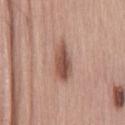The lesion was photographed on a routine skin check and not biopsied; there is no pathology result. From the lower back. The tile uses white-light illumination. The patient is a male about 45 years old. An algorithmic analysis of the crop reported an area of roughly 9.5 mm² and a symmetry-axis asymmetry near 0.2. It also reported roughly 13 lightness units darker than nearby skin and a normalized lesion–skin contrast near 9. The analysis additionally found an automated nevus-likeness rating near 95 out of 100 and a detector confidence of about 100 out of 100 that the crop contains a lesion. A 15 mm crop from a total-body photograph taken for skin-cancer surveillance.